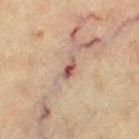Imaged during a routine full-body skin examination; the lesion was not biopsied and no histopathology is available. A 15 mm close-up extracted from a 3D total-body photography capture. Imaged with cross-polarized lighting. A female subject, aged around 65. The lesion is on the leg. An algorithmic analysis of the crop reported a border-irregularity index near 3.5/10, a color-variation rating of about 2/10, and a peripheral color-asymmetry measure near 0. The software also gave a classifier nevus-likeness of about 0/100 and a detector confidence of about 100 out of 100 that the crop contains a lesion.